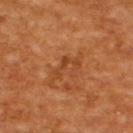Case summary:
* follow-up · imaged on a skin check; not biopsied